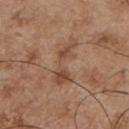Impression:
Recorded during total-body skin imaging; not selected for excision or biopsy.
Clinical summary:
The lesion is on the chest. A 15 mm close-up tile from a total-body photography series done for melanoma screening. The subject is a male approximately 55 years of age.